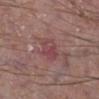Assessment:
Captured during whole-body skin photography for melanoma surveillance; the lesion was not biopsied.
Acquisition and patient details:
A 15 mm crop from a total-body photograph taken for skin-cancer surveillance. The lesion is on the right lower leg. A male patient about 65 years old. The lesion-visualizer software estimated a footprint of about 6.5 mm² and two-axis asymmetry of about 0.25. It also reported about 7 CIELAB-L* units darker than the surrounding skin and a normalized border contrast of about 5.5. And it measured an automated nevus-likeness rating near 0 out of 100 and lesion-presence confidence of about 100/100.